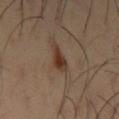| feature | finding |
|---|---|
| imaging modality | ~15 mm crop, total-body skin-cancer survey |
| lighting | cross-polarized illumination |
| location | the left upper arm |
| size | about 3 mm |
| patient | male, aged 38 to 42 |